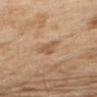Clinical impression: Imaged during a routine full-body skin examination; the lesion was not biopsied and no histopathology is available. Context: A male patient, roughly 70 years of age. This image is a 15 mm lesion crop taken from a total-body photograph. From the upper back.